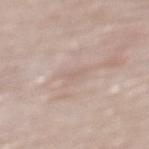Assessment: This lesion was catalogued during total-body skin photography and was not selected for biopsy. Context: Located on the upper back. The tile uses white-light illumination. The subject is a male approximately 85 years of age or older. The recorded lesion diameter is about 2.5 mm. Automated image analysis of the tile measured an automated nevus-likeness rating near 0 out of 100 and a detector confidence of about 15 out of 100 that the crop contains a lesion. A lesion tile, about 15 mm wide, cut from a 3D total-body photograph.